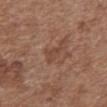Findings:
* follow-up · catalogued during a skin exam; not biopsied
* body site · the chest
* imaging modality · total-body-photography crop, ~15 mm field of view
* illumination · white-light
* subject · female, aged 73–77
* diameter · about 3 mm